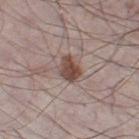illumination — white-light | automated metrics — a footprint of about 6.5 mm², an outline eccentricity of about 0.6 (0 = round, 1 = elongated), and a symmetry-axis asymmetry near 0.15; a mean CIELAB color near L≈47 a*≈17 b*≈23 and about 13 CIELAB-L* units darker than the surrounding skin; a classifier nevus-likeness of about 95/100 and a lesion-detection confidence of about 100/100 | acquisition — ~15 mm crop, total-body skin-cancer survey | body site — the left thigh | lesion size — about 3 mm | patient — male, aged 63–67.